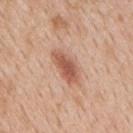Clinical impression:
The lesion was photographed on a routine skin check and not biopsied; there is no pathology result.
Image and clinical context:
The lesion is on the mid back. Imaged with white-light lighting. A male patient, in their mid- to late 60s. Cropped from a total-body skin-imaging series; the visible field is about 15 mm. Longest diameter approximately 4.5 mm. The lesion-visualizer software estimated about 12 CIELAB-L* units darker than the surrounding skin. The software also gave a nevus-likeness score of about 95/100 and a detector confidence of about 100 out of 100 that the crop contains a lesion.